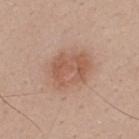Imaged during a routine full-body skin examination; the lesion was not biopsied and no histopathology is available.
A male subject aged around 40.
Cropped from a total-body skin-imaging series; the visible field is about 15 mm.
Located on the upper back.